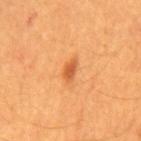- notes · no biopsy performed (imaged during a skin exam)
- subject · male, roughly 60 years of age
- body site · the back
- image · ~15 mm crop, total-body skin-cancer survey